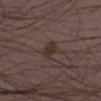The lesion was tiled from a total-body skin photograph and was not biopsied.
Captured under white-light illumination.
The lesion is on the left lower leg.
The subject is a male aged 38–42.
A close-up tile cropped from a whole-body skin photograph, about 15 mm across.